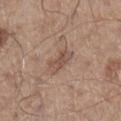Part of a total-body skin-imaging series; this lesion was reviewed on a skin check and was not flagged for biopsy. Captured under white-light illumination. Measured at roughly 3 mm in maximum diameter. On the left lower leg. Automated image analysis of the tile measured a lesion area of about 5 mm², an outline eccentricity of about 0.8 (0 = round, 1 = elongated), and a symmetry-axis asymmetry near 0.25. And it measured a border-irregularity index near 3/10 and radial color variation of about 1. The software also gave lesion-presence confidence of about 100/100. A close-up tile cropped from a whole-body skin photograph, about 15 mm across. A male subject, roughly 60 years of age.